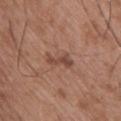Recorded during total-body skin imaging; not selected for excision or biopsy. From the back. This is a white-light tile. Cropped from a whole-body photographic skin survey; the tile spans about 15 mm. A male subject, in their 50s. The total-body-photography lesion software estimated an area of roughly 4 mm² and two-axis asymmetry of about 0.4. And it measured an average lesion color of about L≈46 a*≈21 b*≈26 (CIELAB) and a lesion-to-skin contrast of about 7 (normalized; higher = more distinct).